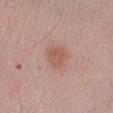| field | value |
|---|---|
| biopsy status | catalogued during a skin exam; not biopsied |
| image source | 15 mm crop, total-body photography |
| lighting | white-light |
| subject | male, approximately 45 years of age |
| site | the left forearm |
| lesion size | about 2.5 mm |